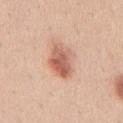Part of a total-body skin-imaging series; this lesion was reviewed on a skin check and was not flagged for biopsy.
On the mid back.
A male patient, aged around 30.
A lesion tile, about 15 mm wide, cut from a 3D total-body photograph.
Imaged with white-light lighting.